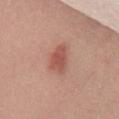{
  "biopsy_status": "not biopsied; imaged during a skin examination"
}